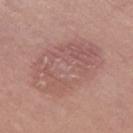Assessment: Captured during whole-body skin photography for melanoma surveillance; the lesion was not biopsied. Background: Longest diameter approximately 8.5 mm. A roughly 15 mm field-of-view crop from a total-body skin photograph. The tile uses white-light illumination. From the left thigh. The patient is a female approximately 65 years of age.